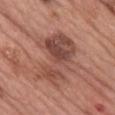lighting: white-light illumination | subject: female, roughly 60 years of age | lesion size: ≈7 mm | anatomic site: the chest | acquisition: ~15 mm crop, total-body skin-cancer survey | automated lesion analysis: a lesion area of about 23 mm² and a shape-asymmetry score of about 0.4 (0 = symmetric); a mean CIELAB color near L≈48 a*≈23 b*≈26, a lesion–skin lightness drop of about 10, and a normalized border contrast of about 7.5; a color-variation rating of about 7/10.